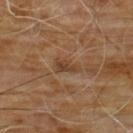notes=total-body-photography surveillance lesion; no biopsy
subject=male, aged 58–62
lighting=cross-polarized illumination
image=~15 mm tile from a whole-body skin photo
body site=the chest
diameter=about 2.5 mm